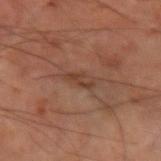| field | value |
|---|---|
| biopsy status | total-body-photography surveillance lesion; no biopsy |
| site | the right forearm |
| lesion diameter | ≈3 mm |
| imaging modality | ~15 mm crop, total-body skin-cancer survey |
| patient | male, aged around 70 |
| illumination | cross-polarized illumination |
| TBP lesion metrics | an area of roughly 3.5 mm², a shape eccentricity near 0.85, and a shape-asymmetry score of about 0.25 (0 = symmetric); a mean CIELAB color near L≈29 a*≈16 b*≈22, about 5 CIELAB-L* units darker than the surrounding skin, and a lesion-to-skin contrast of about 6 (normalized; higher = more distinct); a classifier nevus-likeness of about 0/100 |